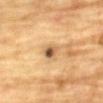Context:
The lesion's longest dimension is about 2.5 mm. This image is a 15 mm lesion crop taken from a total-body photograph. Automated tile analysis of the lesion measured a mean CIELAB color near L≈52 a*≈15 b*≈33, a lesion–skin lightness drop of about 12, and a lesion-to-skin contrast of about 8.5 (normalized; higher = more distinct). The analysis additionally found border irregularity of about 2 on a 0–10 scale, a within-lesion color-variation index near 8.5/10, and peripheral color asymmetry of about 3. The tile uses cross-polarized illumination. From the chest. A male patient aged approximately 85 or older.